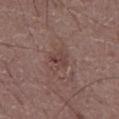Captured during whole-body skin photography for melanoma surveillance; the lesion was not biopsied.
The lesion is located on the abdomen.
Captured under white-light illumination.
The subject is a male aged 58 to 62.
A lesion tile, about 15 mm wide, cut from a 3D total-body photograph.
The recorded lesion diameter is about 2.5 mm.
An algorithmic analysis of the crop reported a footprint of about 3.5 mm², an outline eccentricity of about 0.7 (0 = round, 1 = elongated), and a shape-asymmetry score of about 0.45 (0 = symmetric). It also reported a nevus-likeness score of about 0/100 and a lesion-detection confidence of about 100/100.